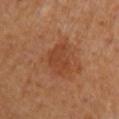biopsy_status: not biopsied; imaged during a skin examination
lesion_size:
  long_diameter_mm_approx: 4.0
image:
  source: total-body photography crop
  field_of_view_mm: 15
site: upper back
patient:
  sex: female
automated_metrics:
  area_mm2_approx: 8.5
  eccentricity: 0.7
  shape_asymmetry: 0.3
  cielab_L: 44
  cielab_a: 26
  cielab_b: 35
  vs_skin_darker_L: 7.0
  vs_skin_contrast_norm: 5.5
  border_irregularity_0_10: 3.0
  color_variation_0_10: 2.5
  peripheral_color_asymmetry: 1.0
  lesion_detection_confidence_0_100: 100
lighting: cross-polarized